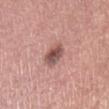| field | value |
|---|---|
| workup | catalogued during a skin exam; not biopsied |
| image source | 15 mm crop, total-body photography |
| size | about 3 mm |
| lighting | white-light |
| site | the leg |
| patient | male, approximately 60 years of age |